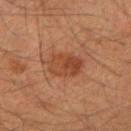{"biopsy_status": "not biopsied; imaged during a skin examination", "patient": {"sex": "male", "age_approx": 35}, "image": {"source": "total-body photography crop", "field_of_view_mm": 15}, "site": "right upper arm"}